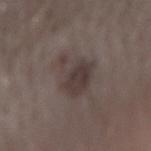Case summary:
• notes · no biopsy performed (imaged during a skin exam)
• anatomic site · the right forearm
• tile lighting · white-light
• patient · male, aged around 30
• size · ~5.5 mm (longest diameter)
• acquisition · ~15 mm tile from a whole-body skin photo